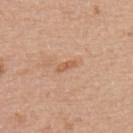From the back.
A female patient, aged around 70.
Cropped from a whole-body photographic skin survey; the tile spans about 15 mm.